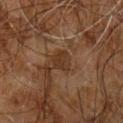<record>
<biopsy_status>not biopsied; imaged during a skin examination</biopsy_status>
<automated_metrics>
  <vs_skin_darker_L>7.0</vs_skin_darker_L>
  <vs_skin_contrast_norm>7.0</vs_skin_contrast_norm>
</automated_metrics>
<patient>
  <sex>male</sex>
  <age_approx>60</age_approx>
</patient>
<lesion_size>
  <long_diameter_mm_approx>2.5</long_diameter_mm_approx>
</lesion_size>
<site>right arm</site>
<lighting>cross-polarized</lighting>
<image>
  <source>total-body photography crop</source>
  <field_of_view_mm>15</field_of_view_mm>
</image>
</record>